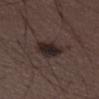Findings:
- patient · male, aged 28 to 32
- site · the right thigh
- imaging modality · total-body-photography crop, ~15 mm field of view